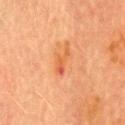Findings:
– biopsy status: catalogued during a skin exam; not biopsied
– patient: male, aged around 70
– image: 15 mm crop, total-body photography
– site: the head or neck